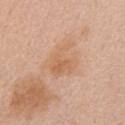<record>
<biopsy_status>not biopsied; imaged during a skin examination</biopsy_status>
<site>mid back</site>
<automated_metrics>
  <cielab_L>63</cielab_L>
  <cielab_a>20</cielab_a>
  <cielab_b>35</cielab_b>
  <vs_skin_contrast_norm>5.5</vs_skin_contrast_norm>
</automated_metrics>
<patient>
  <sex>female</sex>
  <age_approx>65</age_approx>
</patient>
<image>
  <source>total-body photography crop</source>
  <field_of_view_mm>15</field_of_view_mm>
</image>
<lesion_size>
  <long_diameter_mm_approx>4.5</long_diameter_mm_approx>
</lesion_size>
<lighting>white-light</lighting>
</record>